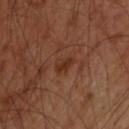image source: ~15 mm tile from a whole-body skin photo | tile lighting: cross-polarized illumination | body site: the upper back | subject: male, approximately 45 years of age | size: ~2.5 mm (longest diameter) | TBP lesion metrics: a lesion area of about 4 mm², a shape eccentricity near 0.75, and two-axis asymmetry of about 0.25; roughly 6 lightness units darker than nearby skin.